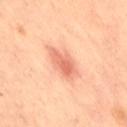  biopsy_status: not biopsied; imaged during a skin examination
  site: lower back
  lighting: cross-polarized
  patient:
    sex: female
    age_approx: 65
  lesion_size:
    long_diameter_mm_approx: 4.0
  image:
    source: total-body photography crop
    field_of_view_mm: 15